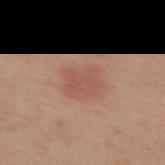• biopsy status: catalogued during a skin exam; not biopsied
• anatomic site: the back
• image source: ~15 mm tile from a whole-body skin photo
• automated lesion analysis: an area of roughly 9 mm², an outline eccentricity of about 0.65 (0 = round, 1 = elongated), and a symmetry-axis asymmetry near 0.2; a mean CIELAB color near L≈54 a*≈23 b*≈29, roughly 7 lightness units darker than nearby skin, and a lesion-to-skin contrast of about 5 (normalized; higher = more distinct); a border-irregularity rating of about 2.5/10, a within-lesion color-variation index near 1.5/10, and a peripheral color-asymmetry measure near 0.5; an automated nevus-likeness rating near 65 out of 100 and lesion-presence confidence of about 100/100
• subject: female, about 40 years old